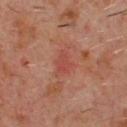lesion size: about 3 mm
anatomic site: the chest
subject: male, aged 58 to 62
image: 15 mm crop, total-body photography